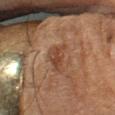Part of a total-body skin-imaging series; this lesion was reviewed on a skin check and was not flagged for biopsy.
A close-up tile cropped from a whole-body skin photograph, about 15 mm across.
A male subject, aged 63–67.
On the left forearm.
Captured under cross-polarized illumination.
Automated image analysis of the tile measured an area of roughly 5.5 mm², an outline eccentricity of about 0.9 (0 = round, 1 = elongated), and two-axis asymmetry of about 0.4. And it measured an average lesion color of about L≈34 a*≈17 b*≈25 (CIELAB) and roughly 7 lightness units darker than nearby skin. And it measured a border-irregularity index near 5/10, a within-lesion color-variation index near 2/10, and a peripheral color-asymmetry measure near 1.